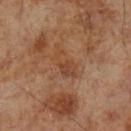follow-up = total-body-photography surveillance lesion; no biopsy
acquisition = ~15 mm tile from a whole-body skin photo
site = the left lower leg
image-analysis metrics = an eccentricity of roughly 0.85 and a symmetry-axis asymmetry near 0.45; border irregularity of about 6 on a 0–10 scale, internal color variation of about 3 on a 0–10 scale, and radial color variation of about 1; a nevus-likeness score of about 0/100 and lesion-presence confidence of about 100/100
lighting = cross-polarized illumination
subject = male, aged around 70